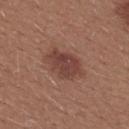This lesion was catalogued during total-body skin photography and was not selected for biopsy. A roughly 15 mm field-of-view crop from a total-body skin photograph. This is a white-light tile. Located on the upper back. A female patient, aged 23 to 27.